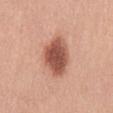This lesion was catalogued during total-body skin photography and was not selected for biopsy.
The lesion's longest dimension is about 5 mm.
The lesion is located on the abdomen.
This is a white-light tile.
The subject is a female in their 40s.
A 15 mm crop from a total-body photograph taken for skin-cancer surveillance.